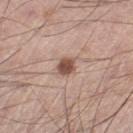Clinical impression:
The lesion was photographed on a routine skin check and not biopsied; there is no pathology result.
Acquisition and patient details:
The lesion-visualizer software estimated an automated nevus-likeness rating near 95 out of 100. A male subject aged 53–57. The lesion is located on the left thigh. Imaged with white-light lighting. A 15 mm crop from a total-body photograph taken for skin-cancer surveillance. The lesion's longest dimension is about 2.5 mm.